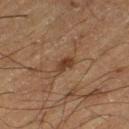{"biopsy_status": "not biopsied; imaged during a skin examination", "lighting": "cross-polarized", "site": "left thigh", "image": {"source": "total-body photography crop", "field_of_view_mm": 15}, "lesion_size": {"long_diameter_mm_approx": 3.0}, "patient": {"sex": "male", "age_approx": 65}}